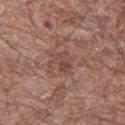| field | value |
|---|---|
| notes | imaged on a skin check; not biopsied |